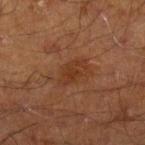Clinical impression: The lesion was photographed on a routine skin check and not biopsied; there is no pathology result. Acquisition and patient details: A roughly 15 mm field-of-view crop from a total-body skin photograph. A male subject, aged 68 to 72. On the right lower leg.